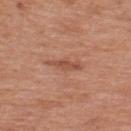The lesion was photographed on a routine skin check and not biopsied; there is no pathology result.
About 3.5 mm across.
The lesion-visualizer software estimated a footprint of about 4 mm², an eccentricity of roughly 0.95, and two-axis asymmetry of about 0.3.
Located on the upper back.
Imaged with white-light lighting.
Cropped from a total-body skin-imaging series; the visible field is about 15 mm.
The subject is a male roughly 70 years of age.